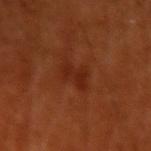Assessment: The lesion was tiled from a total-body skin photograph and was not biopsied. Image and clinical context: The lesion is located on the left upper arm. A roughly 15 mm field-of-view crop from a total-body skin photograph. A male patient aged 68 to 72. Imaged with cross-polarized lighting.